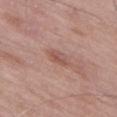Part of a total-body skin-imaging series; this lesion was reviewed on a skin check and was not flagged for biopsy. A region of skin cropped from a whole-body photographic capture, roughly 15 mm wide. The tile uses white-light illumination. Approximately 2.5 mm at its widest. A male subject about 50 years old. Located on the lower back. An algorithmic analysis of the crop reported a lesion area of about 2.5 mm², an eccentricity of roughly 0.85, and two-axis asymmetry of about 0.3. And it measured a nevus-likeness score of about 20/100 and a detector confidence of about 100 out of 100 that the crop contains a lesion.